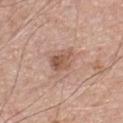* notes — catalogued during a skin exam; not biopsied
* size — ≈3 mm
* lighting — white-light
* body site — the chest
* image source — 15 mm crop, total-body photography
* patient — male, aged around 55